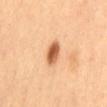Notes:
– notes · total-body-photography surveillance lesion; no biopsy
– imaging modality · 15 mm crop, total-body photography
– body site · the mid back
– tile lighting · cross-polarized
– diameter · ~3 mm (longest diameter)
– subject · male, aged around 40